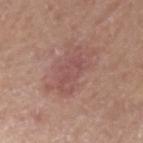follow-up: imaged on a skin check; not biopsied | tile lighting: white-light | image source: 15 mm crop, total-body photography | TBP lesion metrics: a lesion color around L≈51 a*≈23 b*≈22 in CIELAB, a lesion–skin lightness drop of about 7, and a lesion-to-skin contrast of about 5.5 (normalized; higher = more distinct); a border-irregularity index near 5.5/10 and a within-lesion color-variation index near 1.5/10 | site: the arm | subject: female, aged approximately 75.